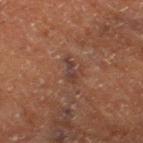Q: Was this lesion biopsied?
A: no biopsy performed (imaged during a skin exam)
Q: Lesion size?
A: about 3 mm
Q: How was this image acquired?
A: total-body-photography crop, ~15 mm field of view
Q: What is the anatomic site?
A: the leg
Q: What are the patient's age and sex?
A: male, roughly 75 years of age
Q: What did automated image analysis measure?
A: a footprint of about 3 mm², a shape eccentricity near 0.9, and a shape-asymmetry score of about 0.35 (0 = symmetric)
Q: How was the tile lit?
A: cross-polarized illumination